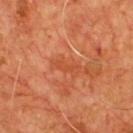Clinical impression:
Recorded during total-body skin imaging; not selected for excision or biopsy.
Clinical summary:
Approximately 3 mm at its widest. The total-body-photography lesion software estimated an average lesion color of about L≈46 a*≈31 b*≈38 (CIELAB) and a normalized border contrast of about 5. A roughly 15 mm field-of-view crop from a total-body skin photograph. A male subject in their mid-50s. Located on the front of the torso.